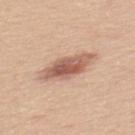Recorded during total-body skin imaging; not selected for excision or biopsy. This image is a 15 mm lesion crop taken from a total-body photograph. From the upper back. The patient is a female aged 43–47.